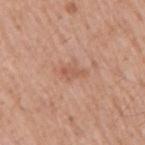Clinical impression: The lesion was tiled from a total-body skin photograph and was not biopsied. Image and clinical context: Captured under white-light illumination. The lesion is on the right upper arm. Approximately 3 mm at its widest. The total-body-photography lesion software estimated an average lesion color of about L≈57 a*≈23 b*≈32 (CIELAB), a lesion–skin lightness drop of about 7, and a lesion-to-skin contrast of about 5.5 (normalized; higher = more distinct). A 15 mm crop from a total-body photograph taken for skin-cancer surveillance. A male subject, aged approximately 55.